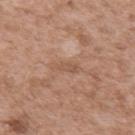{"image": {"source": "total-body photography crop", "field_of_view_mm": 15}, "automated_metrics": {"cielab_L": 54, "cielab_a": 20, "cielab_b": 29, "vs_skin_darker_L": 7.0, "vs_skin_contrast_norm": 5.0}, "site": "arm", "lesion_size": {"long_diameter_mm_approx": 3.0}, "patient": {"sex": "male", "age_approx": 50}, "lighting": "white-light"}